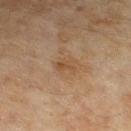biopsy status = imaged on a skin check; not biopsied | patient = female, aged 58 to 62 | site = the left lower leg | tile lighting = cross-polarized illumination | image-analysis metrics = about 6 CIELAB-L* units darker than the surrounding skin and a normalized border contrast of about 5.5 | image = ~15 mm crop, total-body skin-cancer survey | size = ≈2.5 mm.